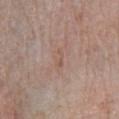Assessment: This lesion was catalogued during total-body skin photography and was not selected for biopsy. Image and clinical context: The lesion-visualizer software estimated a mean CIELAB color near L≈55 a*≈18 b*≈26, about 5 CIELAB-L* units darker than the surrounding skin, and a normalized lesion–skin contrast near 4.5. A male subject in their 60s. Located on the right lower leg. A close-up tile cropped from a whole-body skin photograph, about 15 mm across. The tile uses white-light illumination. Approximately 2.5 mm at its widest.